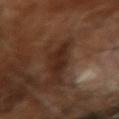follow-up = catalogued during a skin exam; not biopsied
image = ~15 mm tile from a whole-body skin photo
patient = male, approximately 65 years of age